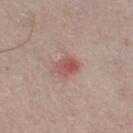The lesion is on the leg. Measured at roughly 3 mm in maximum diameter. Cropped from a whole-body photographic skin survey; the tile spans about 15 mm. A male patient, aged 63–67. This is a white-light tile.